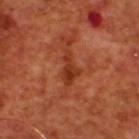Q: Was this lesion biopsied?
A: total-body-photography surveillance lesion; no biopsy
Q: How large is the lesion?
A: ~4 mm (longest diameter)
Q: What is the anatomic site?
A: the upper back
Q: What did automated image analysis measure?
A: a mean CIELAB color near L≈35 a*≈30 b*≈35, about 9 CIELAB-L* units darker than the surrounding skin, and a normalized border contrast of about 7.5; a nevus-likeness score of about 0/100 and a lesion-detection confidence of about 100/100
Q: What are the patient's age and sex?
A: male, roughly 70 years of age
Q: Illumination type?
A: cross-polarized
Q: What kind of image is this?
A: 15 mm crop, total-body photography